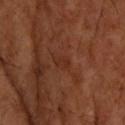workup: imaged on a skin check; not biopsied | subject: male, aged 53 to 57 | body site: the upper back | tile lighting: cross-polarized illumination | imaging modality: ~15 mm tile from a whole-body skin photo.